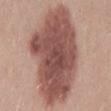- notes — catalogued during a skin exam; not biopsied
- imaging modality — ~15 mm tile from a whole-body skin photo
- site — the back
- patient — male, approximately 25 years of age
- illumination — white-light